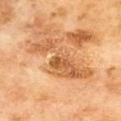follow-up: no biopsy performed (imaged during a skin exam)
tile lighting: cross-polarized
automated lesion analysis: a footprint of about 18 mm², an outline eccentricity of about 0.95 (0 = round, 1 = elongated), and a symmetry-axis asymmetry near 0.5; a classifier nevus-likeness of about 0/100 and a lesion-detection confidence of about 95/100
lesion diameter: about 9 mm
anatomic site: the upper back
subject: male, roughly 70 years of age
image: ~15 mm tile from a whole-body skin photo